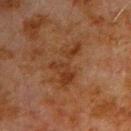Clinical impression: Recorded during total-body skin imaging; not selected for excision or biopsy. Clinical summary: The lesion is on the left upper arm. A male subject, aged 78–82. A 15 mm close-up extracted from a 3D total-body photography capture. Automated image analysis of the tile measured a lesion color around L≈29 a*≈18 b*≈28 in CIELAB, about 6 CIELAB-L* units darker than the surrounding skin, and a lesion-to-skin contrast of about 6.5 (normalized; higher = more distinct). And it measured a border-irregularity rating of about 8.5/10, a within-lesion color-variation index near 3.5/10, and peripheral color asymmetry of about 1. It also reported a nevus-likeness score of about 0/100 and a detector confidence of about 100 out of 100 that the crop contains a lesion. Approximately 6 mm at its widest.